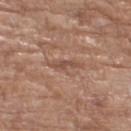Captured during whole-body skin photography for melanoma surveillance; the lesion was not biopsied. Automated tile analysis of the lesion measured a footprint of about 2.5 mm² and two-axis asymmetry of about 0.5. The software also gave an average lesion color of about L≈49 a*≈20 b*≈27 (CIELAB), a lesion–skin lightness drop of about 8, and a lesion-to-skin contrast of about 6 (normalized; higher = more distinct). And it measured border irregularity of about 6 on a 0–10 scale, a within-lesion color-variation index near 0/10, and peripheral color asymmetry of about 0. Cropped from a total-body skin-imaging series; the visible field is about 15 mm. A female patient, aged around 75. Measured at roughly 3 mm in maximum diameter. Located on the right thigh.